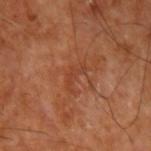This lesion was catalogued during total-body skin photography and was not selected for biopsy.
Measured at roughly 3.5 mm in maximum diameter.
The tile uses cross-polarized illumination.
A lesion tile, about 15 mm wide, cut from a 3D total-body photograph.
A patient in their mid-60s.
The lesion is located on the left upper arm.